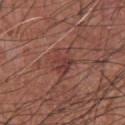imaging modality: total-body-photography crop, ~15 mm field of view
tile lighting: white-light
patient: male, in their mid- to late 70s
body site: the front of the torso
lesion diameter: ~4 mm (longest diameter)